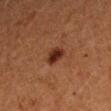• follow-up · no biopsy performed (imaged during a skin exam)
• lesion diameter · about 2.5 mm
• anatomic site · the left forearm
• imaging modality · ~15 mm crop, total-body skin-cancer survey
• illumination · cross-polarized illumination
• subject · female, about 40 years old